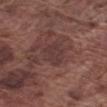Q: Was this lesion biopsied?
A: no biopsy performed (imaged during a skin exam)
Q: What kind of image is this?
A: total-body-photography crop, ~15 mm field of view
Q: Where on the body is the lesion?
A: the right upper arm
Q: Patient demographics?
A: male, approximately 75 years of age
Q: What did automated image analysis measure?
A: a lesion area of about 6.5 mm², a shape eccentricity near 0.75, and two-axis asymmetry of about 0.3; an average lesion color of about L≈36 a*≈19 b*≈19 (CIELAB) and a lesion-to-skin contrast of about 5 (normalized; higher = more distinct); border irregularity of about 3.5 on a 0–10 scale and peripheral color asymmetry of about 0.5; a classifier nevus-likeness of about 0/100 and a lesion-detection confidence of about 95/100